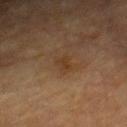{"patient": {"sex": "male", "age_approx": "85+"}, "image": {"source": "total-body photography crop", "field_of_view_mm": 15}, "lesion_size": {"long_diameter_mm_approx": 2.5}, "automated_metrics": {"area_mm2_approx": 3.0, "eccentricity": 0.8, "shape_asymmetry": 0.4, "cielab_L": 31, "cielab_a": 16, "cielab_b": 28, "nevus_likeness_0_100": 0}, "site": "chest"}